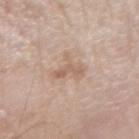The lesion was photographed on a routine skin check and not biopsied; there is no pathology result. The lesion-visualizer software estimated a lesion area of about 6.5 mm², an outline eccentricity of about 0.65 (0 = round, 1 = elongated), and two-axis asymmetry of about 0.6. And it measured a mean CIELAB color near L≈62 a*≈16 b*≈29, about 8 CIELAB-L* units darker than the surrounding skin, and a normalized border contrast of about 5.5. And it measured border irregularity of about 7.5 on a 0–10 scale, a within-lesion color-variation index near 2.5/10, and peripheral color asymmetry of about 1. And it measured a detector confidence of about 100 out of 100 that the crop contains a lesion. A male patient approximately 80 years of age. This is a white-light tile. Measured at roughly 3.5 mm in maximum diameter. On the left forearm. Cropped from a total-body skin-imaging series; the visible field is about 15 mm.